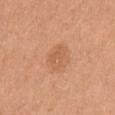biopsy status: total-body-photography surveillance lesion; no biopsy | body site: the front of the torso | acquisition: 15 mm crop, total-body photography | patient: male, aged 63 to 67 | lesion size: about 3 mm | lighting: white-light.